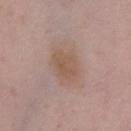This lesion was catalogued during total-body skin photography and was not selected for biopsy. Cropped from a total-body skin-imaging series; the visible field is about 15 mm. A female patient approximately 50 years of age. The lesion is located on the chest. Imaged with white-light lighting. Automated tile analysis of the lesion measured an average lesion color of about L≈54 a*≈16 b*≈27 (CIELAB). The analysis additionally found a border-irregularity rating of about 2/10, a color-variation rating of about 2.5/10, and radial color variation of about 1. It also reported a nevus-likeness score of about 5/100. The lesion's longest dimension is about 3.5 mm.